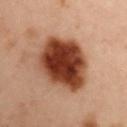Q: Was this lesion biopsied?
A: imaged on a skin check; not biopsied
Q: Lesion size?
A: about 7 mm
Q: Lesion location?
A: the right upper arm
Q: Patient demographics?
A: male, in their mid-50s
Q: What lighting was used for the tile?
A: cross-polarized
Q: What is the imaging modality?
A: total-body-photography crop, ~15 mm field of view